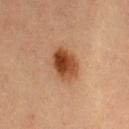This is a cross-polarized tile.
Measured at roughly 4 mm in maximum diameter.
The lesion-visualizer software estimated a footprint of about 11 mm² and a shape eccentricity near 0.55. The software also gave a mean CIELAB color near L≈41 a*≈22 b*≈32, roughly 13 lightness units darker than nearby skin, and a normalized lesion–skin contrast near 10.5.
A female subject about 70 years old.
From the left thigh.
A close-up tile cropped from a whole-body skin photograph, about 15 mm across.